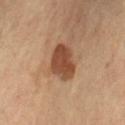This lesion was catalogued during total-body skin photography and was not selected for biopsy.
A 15 mm crop from a total-body photograph taken for skin-cancer surveillance.
About 4.5 mm across.
The patient is a female about 70 years old.
Automated tile analysis of the lesion measured an eccentricity of roughly 0.75.
From the right thigh.
Captured under cross-polarized illumination.